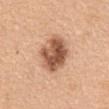biopsy_status: not biopsied; imaged during a skin examination
lighting: white-light
patient:
  sex: female
  age_approx: 55
image:
  source: total-body photography crop
  field_of_view_mm: 15
site: front of the torso
lesion_size:
  long_diameter_mm_approx: 5.0